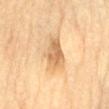The lesion was tiled from a total-body skin photograph and was not biopsied. A female patient aged approximately 80. A close-up tile cropped from a whole-body skin photograph, about 15 mm across. Automated tile analysis of the lesion measured a classifier nevus-likeness of about 25/100 and a lesion-detection confidence of about 100/100. The lesion is on the mid back. The recorded lesion diameter is about 4 mm.